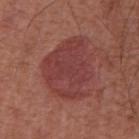<tbp_lesion>
  <biopsy_status>not biopsied; imaged during a skin examination</biopsy_status>
  <image>
    <source>total-body photography crop</source>
    <field_of_view_mm>15</field_of_view_mm>
  </image>
  <site>abdomen</site>
  <lesion_size>
    <long_diameter_mm_approx>7.0</long_diameter_mm_approx>
  </lesion_size>
  <lighting>white-light</lighting>
  <patient>
    <sex>male</sex>
    <age_approx>65</age_approx>
  </patient>
</tbp_lesion>